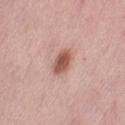No biopsy was performed on this lesion — it was imaged during a full skin examination and was not determined to be concerning. Automated tile analysis of the lesion measured a lesion area of about 5.5 mm² and a shape-asymmetry score of about 0.15 (0 = symmetric). It also reported an average lesion color of about L≈55 a*≈23 b*≈27 (CIELAB), about 14 CIELAB-L* units darker than the surrounding skin, and a lesion-to-skin contrast of about 9.5 (normalized; higher = more distinct). It also reported a classifier nevus-likeness of about 95/100. Longest diameter approximately 3.5 mm. From the leg. A lesion tile, about 15 mm wide, cut from a 3D total-body photograph. Imaged with white-light lighting. A female subject aged approximately 50.